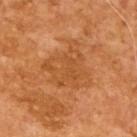Notes:
- notes: total-body-photography surveillance lesion; no biopsy
- lighting: cross-polarized illumination
- subject: male, about 65 years old
- TBP lesion metrics: an average lesion color of about L≈51 a*≈26 b*≈42 (CIELAB), roughly 7 lightness units darker than nearby skin, and a normalized lesion–skin contrast near 5; border irregularity of about 4.5 on a 0–10 scale, a color-variation rating of about 3/10, and radial color variation of about 1
- diameter: ≈6 mm
- image: ~15 mm crop, total-body skin-cancer survey